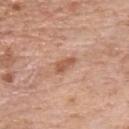location — the chest
lesion size — ≈3 mm
imaging modality — ~15 mm tile from a whole-body skin photo
tile lighting — white-light
subject — male, aged 68 to 72